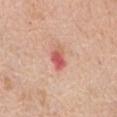follow-up — total-body-photography surveillance lesion; no biopsy | automated lesion analysis — a lesion color around L≈59 a*≈33 b*≈29 in CIELAB and a normalized border contrast of about 8; a within-lesion color-variation index near 6.5/10 and peripheral color asymmetry of about 2.5; a nevus-likeness score of about 0/100 and lesion-presence confidence of about 100/100 | body site — the abdomen | size — about 3 mm | imaging modality — total-body-photography crop, ~15 mm field of view | subject — female, roughly 75 years of age | lighting — white-light.